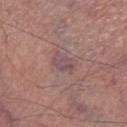Case summary:
* follow-up · total-body-photography surveillance lesion; no biopsy
* TBP lesion metrics · an automated nevus-likeness rating near 0 out of 100 and lesion-presence confidence of about 90/100
* subject · male, aged around 70
* image · ~15 mm tile from a whole-body skin photo
* lesion size · about 3 mm
* anatomic site · the leg
* tile lighting · white-light illumination